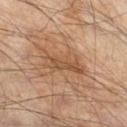Imaged during a routine full-body skin examination; the lesion was not biopsied and no histopathology is available. The recorded lesion diameter is about 5.5 mm. This is a cross-polarized tile. A male patient, aged around 65. This image is a 15 mm lesion crop taken from a total-body photograph. Automated image analysis of the tile measured a shape eccentricity near 0.95 and a symmetry-axis asymmetry near 0.35. The software also gave border irregularity of about 5 on a 0–10 scale and peripheral color asymmetry of about 1. The software also gave an automated nevus-likeness rating near 5 out of 100 and a detector confidence of about 95 out of 100 that the crop contains a lesion. On the right lower leg.